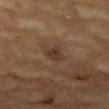Case summary:
* image · 15 mm crop, total-body photography
* subject · male, aged approximately 85 or older
* body site · the mid back
* lesion diameter · about 2.5 mm